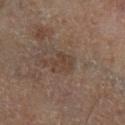Assessment:
Imaged during a routine full-body skin examination; the lesion was not biopsied and no histopathology is available.
Clinical summary:
The tile uses cross-polarized illumination. The subject is a male aged approximately 70. Automated image analysis of the tile measured a lesion area of about 5 mm², a shape eccentricity near 0.65, and two-axis asymmetry of about 0.35. And it measured border irregularity of about 4.5 on a 0–10 scale, internal color variation of about 2 on a 0–10 scale, and a peripheral color-asymmetry measure near 0.5. And it measured an automated nevus-likeness rating near 0 out of 100 and a lesion-detection confidence of about 100/100. Located on the left lower leg. A 15 mm crop from a total-body photograph taken for skin-cancer surveillance.